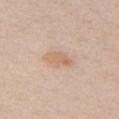The lesion was tiled from a total-body skin photograph and was not biopsied. Automated tile analysis of the lesion measured an average lesion color of about L≈65 a*≈18 b*≈30 (CIELAB). The tile uses white-light illumination. The lesion is located on the chest. This image is a 15 mm lesion crop taken from a total-body photograph. The subject is a female aged 38 to 42.